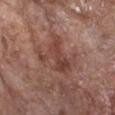Imaged during a routine full-body skin examination; the lesion was not biopsied and no histopathology is available. A female patient in their mid-70s. A roughly 15 mm field-of-view crop from a total-body skin photograph. Located on the front of the torso.